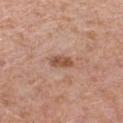follow-up: imaged on a skin check; not biopsied | TBP lesion metrics: a lesion area of about 3.5 mm² and a symmetry-axis asymmetry near 0.3 | image source: ~15 mm tile from a whole-body skin photo | illumination: white-light | anatomic site: the leg | lesion diameter: about 2.5 mm | subject: female, aged around 40.